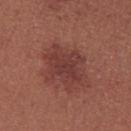Case summary:
• workup — catalogued during a skin exam; not biopsied
• lighting — white-light
• size — about 5 mm
• image source — total-body-photography crop, ~15 mm field of view
• location — the arm
• subject — female, aged 23 to 27